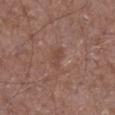Notes:
* follow-up · no biopsy performed (imaged during a skin exam)
* subject · male, aged around 45
* illumination · white-light illumination
* imaging modality · ~15 mm crop, total-body skin-cancer survey
* automated metrics · an eccentricity of roughly 0.85 and a shape-asymmetry score of about 0.4 (0 = symmetric); a border-irregularity index near 4/10 and peripheral color asymmetry of about 0; an automated nevus-likeness rating near 0 out of 100 and a detector confidence of about 100 out of 100 that the crop contains a lesion
* location · the leg
* lesion size · about 2.5 mm